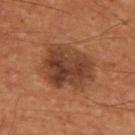– follow-up · imaged on a skin check; not biopsied
– image source · ~15 mm crop, total-body skin-cancer survey
– site · the upper back
– patient · male, aged around 55